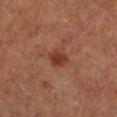workup: imaged on a skin check; not biopsied.